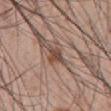| field | value |
|---|---|
| follow-up | catalogued during a skin exam; not biopsied |
| image source | ~15 mm crop, total-body skin-cancer survey |
| subject | male, aged around 45 |
| site | the front of the torso |
| lesion size | ≈2.5 mm |
| automated metrics | a footprint of about 4 mm² and two-axis asymmetry of about 0.35; a border-irregularity index near 3.5/10, internal color variation of about 4 on a 0–10 scale, and radial color variation of about 1.5; a classifier nevus-likeness of about 0/100 and lesion-presence confidence of about 100/100 |
| tile lighting | white-light |